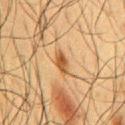Part of a total-body skin-imaging series; this lesion was reviewed on a skin check and was not flagged for biopsy.
This image is a 15 mm lesion crop taken from a total-body photograph.
From the chest.
The subject is a male approximately 60 years of age.
An algorithmic analysis of the crop reported a footprint of about 4 mm² and a shape eccentricity near 0.9. And it measured a mean CIELAB color near L≈44 a*≈18 b*≈34, roughly 10 lightness units darker than nearby skin, and a normalized border contrast of about 8.5. The software also gave a border-irregularity index near 2/10, a color-variation rating of about 4.5/10, and radial color variation of about 1.5. And it measured an automated nevus-likeness rating near 90 out of 100 and lesion-presence confidence of about 100/100.
Measured at roughly 3 mm in maximum diameter.
Captured under cross-polarized illumination.